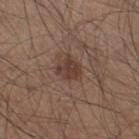The lesion was photographed on a routine skin check and not biopsied; there is no pathology result.
The subject is a male aged 43 to 47.
The lesion is on the right thigh.
A lesion tile, about 15 mm wide, cut from a 3D total-body photograph.